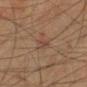This lesion was catalogued during total-body skin photography and was not selected for biopsy. From the left lower leg. A 15 mm crop from a total-body photograph taken for skin-cancer surveillance. Automated image analysis of the tile measured a shape eccentricity near 0.9 and a shape-asymmetry score of about 0.35 (0 = symmetric). The analysis additionally found about 6 CIELAB-L* units darker than the surrounding skin and a normalized lesion–skin contrast near 5.5. This is a cross-polarized tile. The subject is a male roughly 70 years of age.